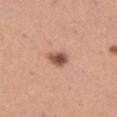Automated image analysis of the tile measured an average lesion color of about L≈53 a*≈24 b*≈29 (CIELAB), about 16 CIELAB-L* units darker than the surrounding skin, and a normalized border contrast of about 10. It also reported a border-irregularity rating of about 2/10 and a within-lesion color-variation index near 6/10. A female patient, roughly 30 years of age. This is a white-light tile. Cropped from a total-body skin-imaging series; the visible field is about 15 mm. On the left thigh. Measured at roughly 2.5 mm in maximum diameter.